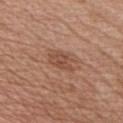<record>
  <biopsy_status>not biopsied; imaged during a skin examination</biopsy_status>
  <patient>
    <sex>male</sex>
    <age_approx>55</age_approx>
  </patient>
  <site>chest</site>
  <image>
    <source>total-body photography crop</source>
    <field_of_view_mm>15</field_of_view_mm>
  </image>
</record>